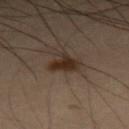Recorded during total-body skin imaging; not selected for excision or biopsy. Located on the leg. A male patient, aged around 55. The lesion's longest dimension is about 3 mm. A close-up tile cropped from a whole-body skin photograph, about 15 mm across. An algorithmic analysis of the crop reported a lesion area of about 5 mm², an eccentricity of roughly 0.45, and a shape-asymmetry score of about 0.35 (0 = symmetric). The software also gave an average lesion color of about L≈21 a*≈12 b*≈20 (CIELAB). And it measured a classifier nevus-likeness of about 95/100 and a detector confidence of about 100 out of 100 that the crop contains a lesion.